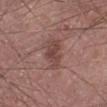- notes: no biopsy performed (imaged during a skin exam)
- subject: male, aged 58–62
- acquisition: ~15 mm tile from a whole-body skin photo
- TBP lesion metrics: a mean CIELAB color near L≈45 a*≈20 b*≈22, about 8 CIELAB-L* units darker than the surrounding skin, and a lesion-to-skin contrast of about 6.5 (normalized; higher = more distinct); border irregularity of about 3 on a 0–10 scale and radial color variation of about 1
- site: the left lower leg
- illumination: white-light illumination
- diameter: ~4 mm (longest diameter)